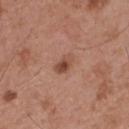biopsy status: no biopsy performed (imaged during a skin exam) | location: the back | image source: total-body-photography crop, ~15 mm field of view | lesion size: ≈2.5 mm | tile lighting: white-light | automated metrics: an outline eccentricity of about 0.75 (0 = round, 1 = elongated); about 10 CIELAB-L* units darker than the surrounding skin and a normalized lesion–skin contrast near 7.5; a border-irregularity index near 2/10 | subject: male, in their mid-50s.